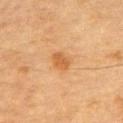follow-up = total-body-photography surveillance lesion; no biopsy
location = the chest
patient = male, roughly 85 years of age or older
image = 15 mm crop, total-body photography